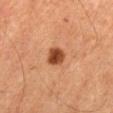Clinical impression:
The lesion was tiled from a total-body skin photograph and was not biopsied.
Acquisition and patient details:
The lesion's longest dimension is about 2.5 mm. The lesion is on the abdomen. The patient is a male aged approximately 65. Imaged with cross-polarized lighting. A 15 mm crop from a total-body photograph taken for skin-cancer surveillance.